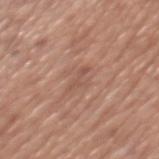workup = total-body-photography surveillance lesion; no biopsy
anatomic site = the mid back
subject = male, aged approximately 65
image = 15 mm crop, total-body photography